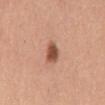biopsy status — total-body-photography surveillance lesion; no biopsy
site — the mid back
subject — male, aged 48 to 52
illumination — white-light
image source — ~15 mm tile from a whole-body skin photo
lesion size — about 3 mm